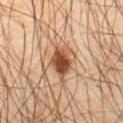Part of a total-body skin-imaging series; this lesion was reviewed on a skin check and was not flagged for biopsy.
A male subject, approximately 65 years of age.
The lesion is on the abdomen.
Longest diameter approximately 3.5 mm.
The tile uses cross-polarized illumination.
The total-body-photography lesion software estimated a lesion color around L≈40 a*≈19 b*≈29 in CIELAB, about 15 CIELAB-L* units darker than the surrounding skin, and a lesion-to-skin contrast of about 12 (normalized; higher = more distinct).
A lesion tile, about 15 mm wide, cut from a 3D total-body photograph.